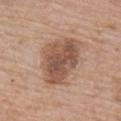This lesion was catalogued during total-body skin photography and was not selected for biopsy.
On the right upper arm.
Automated tile analysis of the lesion measured a footprint of about 22 mm² and a symmetry-axis asymmetry near 0.2. The analysis additionally found a lesion color around L≈53 a*≈19 b*≈28 in CIELAB, roughly 12 lightness units darker than nearby skin, and a normalized lesion–skin contrast near 8.5. The software also gave a border-irregularity rating of about 2.5/10, internal color variation of about 5 on a 0–10 scale, and radial color variation of about 1.5.
A male patient, aged approximately 60.
Cropped from a whole-body photographic skin survey; the tile spans about 15 mm.
The recorded lesion diameter is about 6.5 mm.